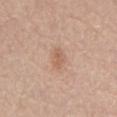The lesion was tiled from a total-body skin photograph and was not biopsied. A male patient, aged 78–82. The lesion is on the mid back. Automated image analysis of the tile measured an area of roughly 4.5 mm², an outline eccentricity of about 0.75 (0 = round, 1 = elongated), and two-axis asymmetry of about 0.25. It also reported a border-irregularity index near 2.5/10, a color-variation rating of about 2/10, and peripheral color asymmetry of about 0.5. A 15 mm crop from a total-body photograph taken for skin-cancer surveillance.